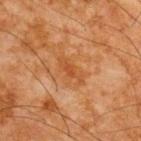Q: Is there a histopathology result?
A: imaged on a skin check; not biopsied
Q: Lesion size?
A: about 4 mm
Q: What kind of image is this?
A: total-body-photography crop, ~15 mm field of view
Q: Illumination type?
A: cross-polarized illumination
Q: What is the anatomic site?
A: the upper back
Q: What are the patient's age and sex?
A: male, in their mid- to late 60s